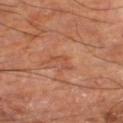Clinical impression: Recorded during total-body skin imaging; not selected for excision or biopsy. Image and clinical context: Located on the right thigh. A patient aged 63 to 67. A close-up tile cropped from a whole-body skin photograph, about 15 mm across. Measured at roughly 2.5 mm in maximum diameter. The tile uses cross-polarized illumination.